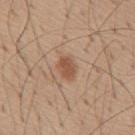{
  "biopsy_status": "not biopsied; imaged during a skin examination",
  "site": "back",
  "lesion_size": {
    "long_diameter_mm_approx": 3.0
  },
  "image": {
    "source": "total-body photography crop",
    "field_of_view_mm": 15
  },
  "lighting": "white-light",
  "patient": {
    "sex": "male",
    "age_approx": 55
  }
}